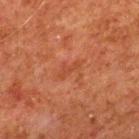imaging modality=~15 mm tile from a whole-body skin photo
patient=male, in their 80s
site=the upper back